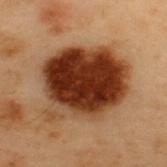Q: Was this lesion biopsied?
A: imaged on a skin check; not biopsied
Q: What lighting was used for the tile?
A: cross-polarized
Q: Who is the patient?
A: male, about 55 years old
Q: What is the imaging modality?
A: ~15 mm crop, total-body skin-cancer survey
Q: How large is the lesion?
A: ≈8 mm
Q: Automated lesion metrics?
A: an average lesion color of about L≈28 a*≈21 b*≈28 (CIELAB) and a lesion-to-skin contrast of about 16 (normalized; higher = more distinct); a border-irregularity index near 2/10, a within-lesion color-variation index near 8/10, and a peripheral color-asymmetry measure near 2.5; a nevus-likeness score of about 100/100
Q: Lesion location?
A: the upper back